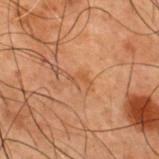The lesion was tiled from a total-body skin photograph and was not biopsied. The patient is a male aged approximately 50. This is a cross-polarized tile. Located on the back. A 15 mm crop from a total-body photograph taken for skin-cancer surveillance. Approximately 3 mm at its widest.